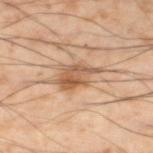Q: Was a biopsy performed?
A: total-body-photography surveillance lesion; no biopsy